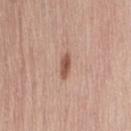Clinical summary: The lesion's longest dimension is about 3 mm. A 15 mm close-up tile from a total-body photography series done for melanoma screening. Located on the lower back. The subject is a female about 65 years old.